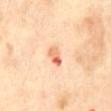Case summary:
- biopsy status — total-body-photography surveillance lesion; no biopsy
- image — ~15 mm tile from a whole-body skin photo
- diameter — ~3 mm (longest diameter)
- patient — male, aged 68 to 72
- automated lesion analysis — a lesion area of about 3.5 mm², a shape eccentricity near 0.85, and a symmetry-axis asymmetry near 0.2
- location — the abdomen